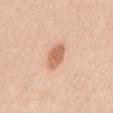notes: catalogued during a skin exam; not biopsied | anatomic site: the mid back | subject: female, about 40 years old | lighting: white-light | automated lesion analysis: a footprint of about 6 mm², an outline eccentricity of about 0.75 (0 = round, 1 = elongated), and a shape-asymmetry score of about 0.15 (0 = symmetric); a mean CIELAB color near L≈64 a*≈23 b*≈34, a lesion–skin lightness drop of about 13, and a normalized border contrast of about 8; border irregularity of about 1.5 on a 0–10 scale and a within-lesion color-variation index near 2.5/10; an automated nevus-likeness rating near 95 out of 100 and a lesion-detection confidence of about 100/100 | image: 15 mm crop, total-body photography | size: ≈3.5 mm.